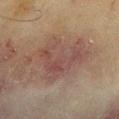Notes:
• workup · catalogued during a skin exam; not biopsied
• site · the arm
• tile lighting · cross-polarized illumination
• acquisition · ~15 mm tile from a whole-body skin photo
• patient · male, aged approximately 70